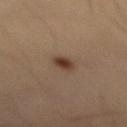Background:
Approximately 2.5 mm at its widest. A close-up tile cropped from a whole-body skin photograph, about 15 mm across. A male patient, aged 53–57. The lesion is located on the mid back.